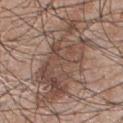No biopsy was performed on this lesion — it was imaged during a full skin examination and was not determined to be concerning. A 15 mm close-up tile from a total-body photography series done for melanoma screening. Captured under white-light illumination. A male patient, aged approximately 45. The lesion is located on the chest. The lesion-visualizer software estimated a lesion color around L≈48 a*≈16 b*≈24 in CIELAB, about 10 CIELAB-L* units darker than the surrounding skin, and a normalized border contrast of about 7.5. The software also gave a border-irregularity rating of about 5/10, a color-variation rating of about 6.5/10, and a peripheral color-asymmetry measure near 2.5. The analysis additionally found a nevus-likeness score of about 5/100 and lesion-presence confidence of about 65/100.